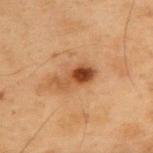{
  "biopsy_status": "not biopsied; imaged during a skin examination"
}